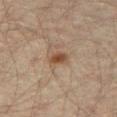Q: Was this lesion biopsied?
A: catalogued during a skin exam; not biopsied
Q: What kind of image is this?
A: ~15 mm tile from a whole-body skin photo
Q: Where on the body is the lesion?
A: the right thigh
Q: Patient demographics?
A: male, aged approximately 45